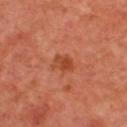The lesion was photographed on a routine skin check and not biopsied; there is no pathology result. Located on the back. A lesion tile, about 15 mm wide, cut from a 3D total-body photograph. A male patient, aged 63 to 67. Approximately 3 mm at its widest. Imaged with cross-polarized lighting.